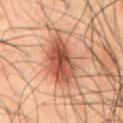The lesion was photographed on a routine skin check and not biopsied; there is no pathology result. The lesion is on the abdomen. The patient is a male aged approximately 50. Automated image analysis of the tile measured border irregularity of about 2 on a 0–10 scale, internal color variation of about 5 on a 0–10 scale, and peripheral color asymmetry of about 1.5. It also reported an automated nevus-likeness rating near 95 out of 100 and a lesion-detection confidence of about 100/100. Cropped from a whole-body photographic skin survey; the tile spans about 15 mm.